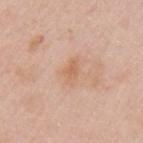Q: Is there a histopathology result?
A: total-body-photography surveillance lesion; no biopsy
Q: What is the imaging modality?
A: total-body-photography crop, ~15 mm field of view
Q: Lesion location?
A: the left upper arm
Q: Patient demographics?
A: female, aged 38–42
Q: How was the tile lit?
A: white-light
Q: What is the lesion's diameter?
A: about 3 mm